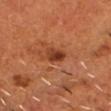Q: What lighting was used for the tile?
A: cross-polarized
Q: How was this image acquired?
A: ~15 mm tile from a whole-body skin photo
Q: Where on the body is the lesion?
A: the head or neck
Q: Who is the patient?
A: male, roughly 55 years of age
Q: Lesion size?
A: about 2.5 mm